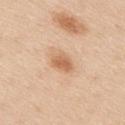Q: Is there a histopathology result?
A: catalogued during a skin exam; not biopsied
Q: Who is the patient?
A: male, aged 33 to 37
Q: What lighting was used for the tile?
A: white-light
Q: What kind of image is this?
A: total-body-photography crop, ~15 mm field of view
Q: What is the anatomic site?
A: the upper back
Q: How large is the lesion?
A: about 3 mm
Q: What did automated image analysis measure?
A: roughly 11 lightness units darker than nearby skin and a normalized border contrast of about 7; a nevus-likeness score of about 95/100 and a detector confidence of about 100 out of 100 that the crop contains a lesion